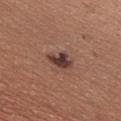Q: Is there a histopathology result?
A: catalogued during a skin exam; not biopsied